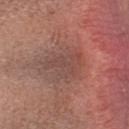- follow-up · no biopsy performed (imaged during a skin exam)
- site · the head or neck
- image-analysis metrics · an average lesion color of about L≈47 a*≈21 b*≈24 (CIELAB), about 6 CIELAB-L* units darker than the surrounding skin, and a normalized lesion–skin contrast near 5; a classifier nevus-likeness of about 0/100 and a lesion-detection confidence of about 95/100
- patient · female, in their mid-30s
- image · total-body-photography crop, ~15 mm field of view
- illumination · white-light
- lesion size · ≈5 mm